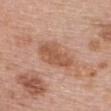<tbp_lesion>
  <biopsy_status>not biopsied; imaged during a skin examination</biopsy_status>
</tbp_lesion>